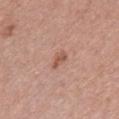diameter — ~2.5 mm (longest diameter)
location — the chest
patient — female, approximately 45 years of age
lighting — white-light
image source — ~15 mm tile from a whole-body skin photo